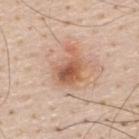Measured at roughly 4.5 mm in maximum diameter. A male subject, approximately 60 years of age. Captured under white-light illumination. A lesion tile, about 15 mm wide, cut from a 3D total-body photograph. Automated image analysis of the tile measured a border-irregularity index near 5.5/10, a within-lesion color-variation index near 6.5/10, and peripheral color asymmetry of about 2. The lesion is on the back.